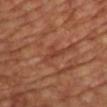Impression:
This lesion was catalogued during total-body skin photography and was not selected for biopsy.
Clinical summary:
On the chest. Cropped from a whole-body photographic skin survey; the tile spans about 15 mm. Imaged with cross-polarized lighting. Longest diameter approximately 2.5 mm. A male subject aged around 55.